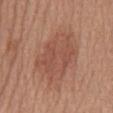Clinical impression:
The lesion was tiled from a total-body skin photograph and was not biopsied.
Background:
A male subject in their mid-60s. Approximately 7 mm at its widest. This is a white-light tile. A close-up tile cropped from a whole-body skin photograph, about 15 mm across. The lesion is located on the front of the torso.